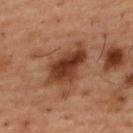<case>
<biopsy_status>not biopsied; imaged during a skin examination</biopsy_status>
<patient>
  <sex>male</sex>
  <age_approx>55</age_approx>
</patient>
<site>upper back</site>
<image>
  <source>total-body photography crop</source>
  <field_of_view_mm>15</field_of_view_mm>
</image>
<lesion_size>
  <long_diameter_mm_approx>6.0</long_diameter_mm_approx>
</lesion_size>
<automated_metrics>
  <area_mm2_approx>16.0</area_mm2_approx>
  <shape_asymmetry>0.25</shape_asymmetry>
  <cielab_L>38</cielab_L>
  <cielab_a>23</cielab_a>
  <cielab_b>30</cielab_b>
  <vs_skin_darker_L>13.0</vs_skin_darker_L>
  <vs_skin_contrast_norm>10.5</vs_skin_contrast_norm>
</automated_metrics>
</case>